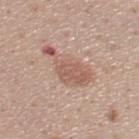Captured during whole-body skin photography for melanoma surveillance; the lesion was not biopsied.
A male patient in their 40s.
The lesion is located on the back.
A close-up tile cropped from a whole-body skin photograph, about 15 mm across.